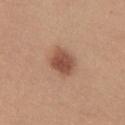follow-up = total-body-photography surveillance lesion; no biopsy | image-analysis metrics = a normalized lesion–skin contrast near 9; an automated nevus-likeness rating near 100 out of 100 and lesion-presence confidence of about 100/100 | site = the chest | subject = female, aged around 45 | image source = ~15 mm crop, total-body skin-cancer survey | illumination = white-light illumination | lesion size = ~3.5 mm (longest diameter).